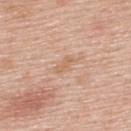Clinical impression: This lesion was catalogued during total-body skin photography and was not selected for biopsy. Clinical summary: Measured at roughly 3 mm in maximum diameter. Cropped from a whole-body photographic skin survey; the tile spans about 15 mm. The total-body-photography lesion software estimated a border-irregularity rating of about 4/10, internal color variation of about 0.5 on a 0–10 scale, and peripheral color asymmetry of about 0. It also reported a classifier nevus-likeness of about 0/100. The subject is a male in their mid- to late 50s. Located on the upper back. Imaged with white-light lighting.